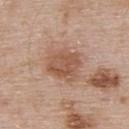workup=imaged on a skin check; not biopsied | patient=male, about 55 years old | acquisition=15 mm crop, total-body photography | site=the upper back | tile lighting=white-light | automated metrics=a lesion area of about 12 mm², a shape eccentricity near 0.55, and a symmetry-axis asymmetry near 0.2; a mean CIELAB color near L≈54 a*≈20 b*≈30, about 10 CIELAB-L* units darker than the surrounding skin, and a normalized border contrast of about 7; a border-irregularity rating of about 2/10, internal color variation of about 4 on a 0–10 scale, and peripheral color asymmetry of about 1.5; a classifier nevus-likeness of about 5/100.